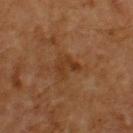| field | value |
|---|---|
| notes | no biopsy performed (imaged during a skin exam) |
| TBP lesion metrics | an outline eccentricity of about 0.7 (0 = round, 1 = elongated); border irregularity of about 5 on a 0–10 scale and a color-variation rating of about 1/10 |
| lesion diameter | ≈3 mm |
| site | the upper back |
| acquisition | total-body-photography crop, ~15 mm field of view |
| illumination | cross-polarized illumination |
| subject | male, aged around 60 |